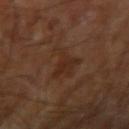  biopsy_status: not biopsied; imaged during a skin examination
  automated_metrics:
    vs_skin_darker_L: 5.0
    vs_skin_contrast_norm: 6.5
    nevus_likeness_0_100: 0
    lesion_detection_confidence_0_100: 100
  site: arm
  image:
    source: total-body photography crop
    field_of_view_mm: 15
  lighting: cross-polarized
  patient:
    sex: male
    age_approx: 70
  lesion_size:
    long_diameter_mm_approx: 5.0